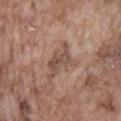biopsy status = imaged on a skin check; not biopsied
site = the abdomen
lesion size = about 4 mm
lighting = white-light
image-analysis metrics = an eccentricity of roughly 0.8; a normalized lesion–skin contrast near 7; a color-variation rating of about 3.5/10 and a peripheral color-asymmetry measure near 1.5; an automated nevus-likeness rating near 0 out of 100
image = 15 mm crop, total-body photography
subject = male, in their mid-70s